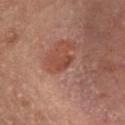workup: total-body-photography surveillance lesion; no biopsy
lighting: cross-polarized illumination
image-analysis metrics: an average lesion color of about L≈37 a*≈22 b*≈25 (CIELAB) and a lesion-to-skin contrast of about 6 (normalized; higher = more distinct); border irregularity of about 3.5 on a 0–10 scale, internal color variation of about 1.5 on a 0–10 scale, and radial color variation of about 0.5
diameter: about 2.5 mm
patient: female, aged around 55
body site: the head or neck
image source: total-body-photography crop, ~15 mm field of view